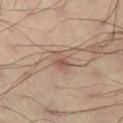notes=imaged on a skin check; not biopsied.